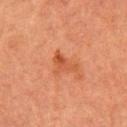Q: Is there a histopathology result?
A: total-body-photography surveillance lesion; no biopsy
Q: What kind of image is this?
A: ~15 mm crop, total-body skin-cancer survey
Q: What is the anatomic site?
A: the right forearm
Q: Who is the patient?
A: female, aged 68–72